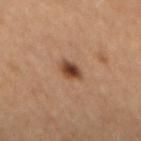The lesion was photographed on a routine skin check and not biopsied; there is no pathology result. From the mid back. A female patient, aged around 60. Measured at roughly 3 mm in maximum diameter. A roughly 15 mm field-of-view crop from a total-body skin photograph.